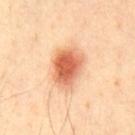Assessment: The lesion was photographed on a routine skin check and not biopsied; there is no pathology result. Context: The lesion is on the chest. A region of skin cropped from a whole-body photographic capture, roughly 15 mm wide. A male subject, roughly 40 years of age.